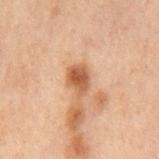Part of a total-body skin-imaging series; this lesion was reviewed on a skin check and was not flagged for biopsy.
The lesion is on the mid back.
A male subject, aged 68–72.
Cropped from a whole-body photographic skin survey; the tile spans about 15 mm.